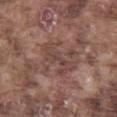Impression:
The lesion was photographed on a routine skin check and not biopsied; there is no pathology result.
Context:
Captured under white-light illumination. The total-body-photography lesion software estimated a footprint of about 9.5 mm² and two-axis asymmetry of about 0.4. And it measured an average lesion color of about L≈44 a*≈19 b*≈22 (CIELAB) and a normalized border contrast of about 6. And it measured border irregularity of about 8 on a 0–10 scale, a color-variation rating of about 2/10, and radial color variation of about 0.5. A 15 mm close-up extracted from a 3D total-body photography capture. A male subject roughly 75 years of age. Approximately 5 mm at its widest. On the left thigh.